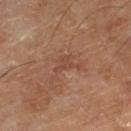Q: Was a biopsy performed?
A: catalogued during a skin exam; not biopsied
Q: How large is the lesion?
A: ~4 mm (longest diameter)
Q: Where on the body is the lesion?
A: the right lower leg
Q: Automated lesion metrics?
A: an average lesion color of about L≈47 a*≈23 b*≈29 (CIELAB), a lesion–skin lightness drop of about 6, and a lesion-to-skin contrast of about 4.5 (normalized; higher = more distinct); border irregularity of about 9 on a 0–10 scale, a color-variation rating of about 1.5/10, and radial color variation of about 0.5; a nevus-likeness score of about 0/100 and a detector confidence of about 100 out of 100 that the crop contains a lesion
Q: How was the tile lit?
A: cross-polarized illumination
Q: What are the patient's age and sex?
A: male, aged approximately 65
Q: How was this image acquired?
A: 15 mm crop, total-body photography